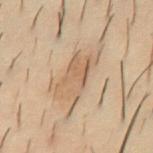follow-up: catalogued during a skin exam; not biopsied
tile lighting: cross-polarized illumination
subject: male, approximately 30 years of age
image-analysis metrics: an outline eccentricity of about 0.8 (0 = round, 1 = elongated) and two-axis asymmetry of about 0.25; a classifier nevus-likeness of about 15/100
body site: the abdomen
size: ~5 mm (longest diameter)
acquisition: total-body-photography crop, ~15 mm field of view